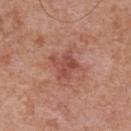Assessment:
Recorded during total-body skin imaging; not selected for excision or biopsy.
Clinical summary:
Longest diameter approximately 3.5 mm. A male subject about 70 years old. Captured under white-light illumination. A region of skin cropped from a whole-body photographic capture, roughly 15 mm wide. On the chest.